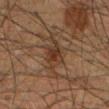The lesion was tiled from a total-body skin photograph and was not biopsied. This image is a 15 mm lesion crop taken from a total-body photograph. The patient is a male about 60 years old. The tile uses cross-polarized illumination. From the mid back.